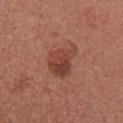Located on the arm. This image is a 15 mm lesion crop taken from a total-body photograph. A female patient in their mid-20s.